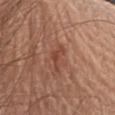biopsy status = total-body-photography surveillance lesion; no biopsy | acquisition = ~15 mm tile from a whole-body skin photo | lesion diameter = about 3.5 mm | automated lesion analysis = a lesion area of about 3.5 mm², a shape eccentricity near 0.95, and two-axis asymmetry of about 0.6; a lesion color around L≈45 a*≈25 b*≈30 in CIELAB, about 8 CIELAB-L* units darker than the surrounding skin, and a normalized border contrast of about 6.5; radial color variation of about 0 | subject = male, roughly 65 years of age | lighting = white-light | anatomic site = the chest.